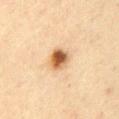– notes — catalogued during a skin exam; not biopsied
– body site — the abdomen
– patient — female, roughly 70 years of age
– tile lighting — cross-polarized
– imaging modality — ~15 mm crop, total-body skin-cancer survey
– lesion size — ≈3 mm